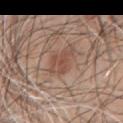Impression: The lesion was tiled from a total-body skin photograph and was not biopsied. Context: From the left upper arm. A lesion tile, about 15 mm wide, cut from a 3D total-body photograph. A male patient about 45 years old. This is a white-light tile.